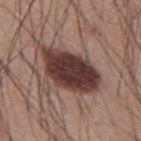notes=imaged on a skin check; not biopsied | tile lighting=white-light illumination | image=~15 mm tile from a whole-body skin photo | size=~7.5 mm (longest diameter) | anatomic site=the mid back | subject=male, in their 60s.